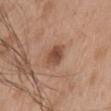Clinical impression:
The lesion was tiled from a total-body skin photograph and was not biopsied.
Clinical summary:
The total-body-photography lesion software estimated a lesion area of about 5.5 mm², a shape eccentricity near 0.75, and a shape-asymmetry score of about 0.3 (0 = symmetric). It also reported an average lesion color of about L≈49 a*≈21 b*≈29 (CIELAB), a lesion–skin lightness drop of about 12, and a normalized lesion–skin contrast near 8.5. The analysis additionally found a border-irregularity index near 3/10, a color-variation rating of about 4/10, and radial color variation of about 1. The lesion is located on the chest. A male patient about 65 years old. This image is a 15 mm lesion crop taken from a total-body photograph. About 3 mm across.